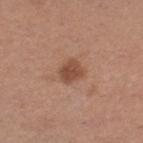Case summary:
* follow-up: catalogued during a skin exam; not biopsied
* body site: the left thigh
* lighting: white-light illumination
* imaging modality: total-body-photography crop, ~15 mm field of view
* patient: female, approximately 30 years of age